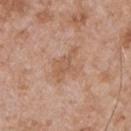This lesion was catalogued during total-body skin photography and was not selected for biopsy. An algorithmic analysis of the crop reported a footprint of about 8 mm², a shape eccentricity near 0.75, and a symmetry-axis asymmetry near 0.35. The software also gave an average lesion color of about L≈57 a*≈20 b*≈31 (CIELAB), about 7 CIELAB-L* units darker than the surrounding skin, and a lesion-to-skin contrast of about 5.5 (normalized; higher = more distinct). It also reported internal color variation of about 2 on a 0–10 scale and peripheral color asymmetry of about 0.5. Measured at roughly 4.5 mm in maximum diameter. Cropped from a whole-body photographic skin survey; the tile spans about 15 mm. This is a white-light tile. The patient is a male aged around 65. The lesion is on the chest.